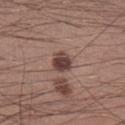biopsy status: no biopsy performed (imaged during a skin exam)
imaging modality: ~15 mm tile from a whole-body skin photo
location: the right lower leg
size: ≈2.5 mm
patient: male, approximately 30 years of age
image-analysis metrics: an area of roughly 6 mm², an eccentricity of roughly 0.3, and two-axis asymmetry of about 0.15; a mean CIELAB color near L≈42 a*≈18 b*≈21, roughly 13 lightness units darker than nearby skin, and a normalized border contrast of about 10.5
tile lighting: white-light illumination